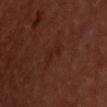The lesion was tiled from a total-body skin photograph and was not biopsied.
A male subject aged 48–52.
Imaged with cross-polarized lighting.
About 3 mm across.
A 15 mm close-up tile from a total-body photography series done for melanoma screening.
Automated image analysis of the tile measured a lesion area of about 3.5 mm², an eccentricity of roughly 0.9, and two-axis asymmetry of about 0.3. The analysis additionally found roughly 4 lightness units darker than nearby skin. And it measured an automated nevus-likeness rating near 5 out of 100 and a detector confidence of about 100 out of 100 that the crop contains a lesion.
The lesion is on the back.